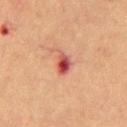- notes: no biopsy performed (imaged during a skin exam)
- subject: male, in their mid- to late 60s
- lighting: cross-polarized
- image source: 15 mm crop, total-body photography
- body site: the mid back
- lesion diameter: ≈3 mm